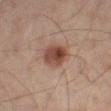Q: Was a biopsy performed?
A: catalogued during a skin exam; not biopsied
Q: What is the anatomic site?
A: the right thigh
Q: Lesion size?
A: about 3.5 mm
Q: Illumination type?
A: cross-polarized illumination
Q: What is the imaging modality?
A: ~15 mm tile from a whole-body skin photo
Q: Patient demographics?
A: male, in their mid- to late 60s